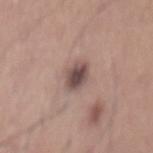Q: Was this lesion biopsied?
A: imaged on a skin check; not biopsied
Q: What did automated image analysis measure?
A: an average lesion color of about L≈49 a*≈17 b*≈19 (CIELAB), a lesion–skin lightness drop of about 14, and a lesion-to-skin contrast of about 10 (normalized; higher = more distinct); a color-variation rating of about 4/10 and radial color variation of about 1
Q: Lesion location?
A: the mid back
Q: What lighting was used for the tile?
A: white-light
Q: How large is the lesion?
A: about 3.5 mm
Q: What are the patient's age and sex?
A: male, about 40 years old
Q: What is the imaging modality?
A: 15 mm crop, total-body photography